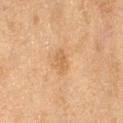This lesion was catalogued during total-body skin photography and was not selected for biopsy.